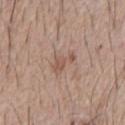| field | value |
|---|---|
| biopsy status | no biopsy performed (imaged during a skin exam) |
| patient | male, aged 63 to 67 |
| acquisition | 15 mm crop, total-body photography |
| lighting | white-light |
| body site | the chest |
| lesion size | ≈3 mm |